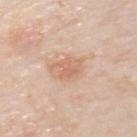biopsy status: catalogued during a skin exam; not biopsied
automated metrics: an outline eccentricity of about 0.5 (0 = round, 1 = elongated) and a symmetry-axis asymmetry near 0.4; a lesion color around L≈65 a*≈20 b*≈32 in CIELAB, a lesion–skin lightness drop of about 8, and a normalized border contrast of about 5.5; a border-irregularity rating of about 5.5/10
illumination: white-light
patient: male, in their mid-70s
diameter: ~3.5 mm (longest diameter)
image source: ~15 mm tile from a whole-body skin photo
body site: the left upper arm